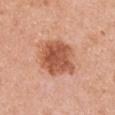{"biopsy_status": "not biopsied; imaged during a skin examination", "lesion_size": {"long_diameter_mm_approx": 6.0}, "site": "arm", "automated_metrics": {"area_mm2_approx": 19.0, "shape_asymmetry": 0.15}, "lighting": "white-light", "image": {"source": "total-body photography crop", "field_of_view_mm": 15}, "patient": {"sex": "female", "age_approx": 50}}